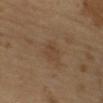{
  "biopsy_status": "not biopsied; imaged during a skin examination",
  "image": {
    "source": "total-body photography crop",
    "field_of_view_mm": 15
  },
  "lighting": "cross-polarized",
  "automated_metrics": {
    "area_mm2_approx": 4.5,
    "eccentricity": 0.65,
    "shape_asymmetry": 0.25,
    "cielab_L": 40,
    "cielab_a": 15,
    "cielab_b": 28,
    "vs_skin_darker_L": 5.0,
    "lesion_detection_confidence_0_100": 100
  },
  "lesion_size": {
    "long_diameter_mm_approx": 3.0
  },
  "patient": {
    "sex": "male",
    "age_approx": 65
  }
}